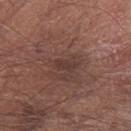This lesion was catalogued during total-body skin photography and was not selected for biopsy.
From the arm.
A male patient aged 73 to 77.
This image is a 15 mm lesion crop taken from a total-body photograph.